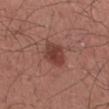Captured during whole-body skin photography for melanoma surveillance; the lesion was not biopsied.
The lesion's longest dimension is about 3.5 mm.
The patient is a male approximately 35 years of age.
This image is a 15 mm lesion crop taken from a total-body photograph.
Located on the arm.